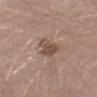Imaged during a routine full-body skin examination; the lesion was not biopsied and no histopathology is available.
From the left thigh.
A male patient about 30 years old.
The tile uses white-light illumination.
A 15 mm close-up extracted from a 3D total-body photography capture.
About 3.5 mm across.